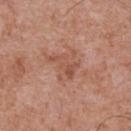Captured during whole-body skin photography for melanoma surveillance; the lesion was not biopsied.
The lesion's longest dimension is about 4 mm.
The lesion is located on the chest.
Cropped from a total-body skin-imaging series; the visible field is about 15 mm.
The total-body-photography lesion software estimated an outline eccentricity of about 0.85 (0 = round, 1 = elongated) and a shape-asymmetry score of about 0.5 (0 = symmetric). The software also gave a nevus-likeness score of about 0/100.
The patient is a male about 70 years old.
Captured under white-light illumination.